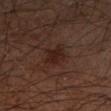Recorded during total-body skin imaging; not selected for excision or biopsy. A lesion tile, about 15 mm wide, cut from a 3D total-body photograph. On the right forearm. The lesion's longest dimension is about 2.5 mm. The subject is a male aged 58–62. Captured under cross-polarized illumination.